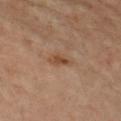The lesion was photographed on a routine skin check and not biopsied; there is no pathology result.